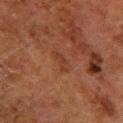<case>
  <automated_metrics>
    <area_mm2_approx>2.5</area_mm2_approx>
    <eccentricity>0.9</eccentricity>
    <shape_asymmetry>0.55</shape_asymmetry>
    <vs_skin_darker_L>5.0</vs_skin_darker_L>
    <vs_skin_contrast_norm>5.0</vs_skin_contrast_norm>
    <border_irregularity_0_10>6.0</border_irregularity_0_10>
    <color_variation_0_10>0.0</color_variation_0_10>
    <peripheral_color_asymmetry>0.0</peripheral_color_asymmetry>
    <nevus_likeness_0_100>0</nevus_likeness_0_100>
  </automated_metrics>
  <lesion_size>
    <long_diameter_mm_approx>3.0</long_diameter_mm_approx>
  </lesion_size>
  <site>left lower leg</site>
  <patient>
    <sex>male</sex>
    <age_approx>80</age_approx>
  </patient>
  <lighting>cross-polarized</lighting>
  <image>
    <source>total-body photography crop</source>
    <field_of_view_mm>15</field_of_view_mm>
  </image>
</case>